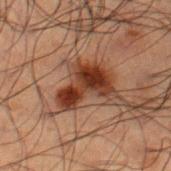Assessment: The lesion was photographed on a routine skin check and not biopsied; there is no pathology result. Context: The lesion is located on the leg. A male patient aged 48–52. The recorded lesion diameter is about 5 mm. This is a cross-polarized tile. Automated image analysis of the tile measured an average lesion color of about L≈27 a*≈20 b*≈25 (CIELAB) and a lesion-to-skin contrast of about 13 (normalized; higher = more distinct). The analysis additionally found a classifier nevus-likeness of about 85/100. A region of skin cropped from a whole-body photographic capture, roughly 15 mm wide.